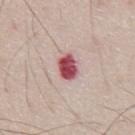Clinical impression: This lesion was catalogued during total-body skin photography and was not selected for biopsy. Context: From the abdomen. A close-up tile cropped from a whole-body skin photograph, about 15 mm across. A male patient aged around 65.